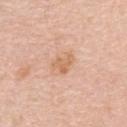follow-up: imaged on a skin check; not biopsied | subject: female, aged 48 to 52 | site: the upper back | image source: ~15 mm crop, total-body skin-cancer survey | automated metrics: a lesion area of about 4 mm², a shape eccentricity near 0.7, and a symmetry-axis asymmetry near 0.5; a lesion color around L≈65 a*≈22 b*≈36 in CIELAB, a lesion–skin lightness drop of about 8, and a lesion-to-skin contrast of about 6.5 (normalized; higher = more distinct); a border-irregularity index near 5/10 and a color-variation rating of about 2/10; lesion-presence confidence of about 100/100.